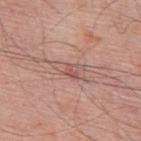The lesion was tiled from a total-body skin photograph and was not biopsied.
The lesion is located on the mid back.
Automated image analysis of the tile measured an outline eccentricity of about 0.9 (0 = round, 1 = elongated) and two-axis asymmetry of about 0.35. And it measured a mean CIELAB color near L≈56 a*≈21 b*≈23 and about 8 CIELAB-L* units darker than the surrounding skin. The software also gave a classifier nevus-likeness of about 0/100 and lesion-presence confidence of about 50/100.
Approximately 4 mm at its widest.
Captured under white-light illumination.
The patient is a male about 60 years old.
A lesion tile, about 15 mm wide, cut from a 3D total-body photograph.